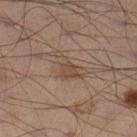This lesion was catalogued during total-body skin photography and was not selected for biopsy.
A male subject, approximately 50 years of age.
A roughly 15 mm field-of-view crop from a total-body skin photograph.
Captured under cross-polarized illumination.
The lesion is located on the left leg.
The recorded lesion diameter is about 4.5 mm.